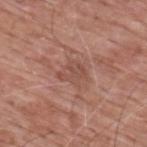workup = no biopsy performed (imaged during a skin exam)
patient = male, aged 58–62
lesion diameter = ≈3.5 mm
imaging modality = total-body-photography crop, ~15 mm field of view
TBP lesion metrics = an average lesion color of about L≈50 a*≈22 b*≈26 (CIELAB), a lesion–skin lightness drop of about 7, and a normalized border contrast of about 5; a border-irregularity index near 4.5/10, a color-variation rating of about 2.5/10, and radial color variation of about 1; an automated nevus-likeness rating near 0 out of 100 and a detector confidence of about 100 out of 100 that the crop contains a lesion
body site = the upper back